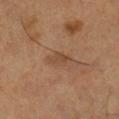notes: catalogued during a skin exam; not biopsied
subject: male, aged around 55
TBP lesion metrics: an area of roughly 4 mm², an eccentricity of roughly 0.85, and a shape-asymmetry score of about 0.3 (0 = symmetric); a lesion color around L≈44 a*≈19 b*≈31 in CIELAB and a normalized lesion–skin contrast near 5.5; a nevus-likeness score of about 0/100 and a detector confidence of about 100 out of 100 that the crop contains a lesion
site: the right lower leg
image source: ~15 mm crop, total-body skin-cancer survey